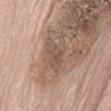Recorded during total-body skin imaging; not selected for excision or biopsy. A region of skin cropped from a whole-body photographic capture, roughly 15 mm wide. This is a white-light tile. A male patient roughly 70 years of age. From the abdomen. The lesion's longest dimension is about 4 mm.